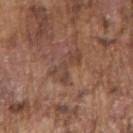Captured during whole-body skin photography for melanoma surveillance; the lesion was not biopsied.
Imaged with white-light lighting.
Cropped from a whole-body photographic skin survey; the tile spans about 15 mm.
A male subject in their mid-70s.
The lesion is on the right upper arm.
Automated image analysis of the tile measured an outline eccentricity of about 0.8 (0 = round, 1 = elongated) and two-axis asymmetry of about 0.3. The software also gave roughly 7 lightness units darker than nearby skin and a lesion-to-skin contrast of about 5.5 (normalized; higher = more distinct). The software also gave a nevus-likeness score of about 0/100 and a lesion-detection confidence of about 90/100.
Approximately 4 mm at its widest.